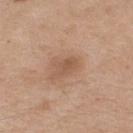workup: total-body-photography surveillance lesion; no biopsy
acquisition: total-body-photography crop, ~15 mm field of view
lighting: white-light
lesion size: ~3 mm (longest diameter)
anatomic site: the upper back
patient: male, in their mid-70s
automated metrics: an area of roughly 4 mm², an eccentricity of roughly 0.75, and a shape-asymmetry score of about 0.3 (0 = symmetric)